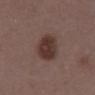The lesion was photographed on a routine skin check and not biopsied; there is no pathology result.
From the mid back.
A 15 mm close-up extracted from a 3D total-body photography capture.
A male subject, in their 50s.
Approximately 5 mm at its widest.
The tile uses white-light illumination.